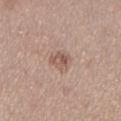The subject is a female aged 38–42. Cropped from a whole-body photographic skin survey; the tile spans about 15 mm. Longest diameter approximately 2.5 mm. Captured under white-light illumination. Located on the left lower leg.